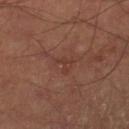Clinical impression:
The lesion was tiled from a total-body skin photograph and was not biopsied.
Context:
A 15 mm close-up tile from a total-body photography series done for melanoma screening. Imaged with cross-polarized lighting. Approximately 2.5 mm at its widest. A male subject about 65 years old. On the right lower leg.